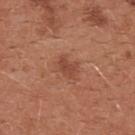notes = total-body-photography surveillance lesion; no biopsy
image = 15 mm crop, total-body photography
subject = female, aged 33–37
anatomic site = the chest